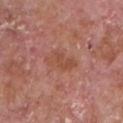lighting: white-light illumination | automated lesion analysis: a footprint of about 7 mm², a shape eccentricity near 0.9, and a shape-asymmetry score of about 0.35 (0 = symmetric); an average lesion color of about L≈49 a*≈25 b*≈30 (CIELAB), roughly 6 lightness units darker than nearby skin, and a lesion-to-skin contrast of about 5.5 (normalized; higher = more distinct) | patient: male, aged approximately 65 | acquisition: total-body-photography crop, ~15 mm field of view | body site: the chest | lesion diameter: about 4.5 mm.